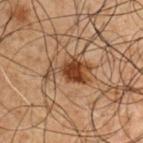Q: Is there a histopathology result?
A: catalogued during a skin exam; not biopsied